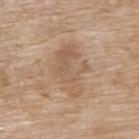TBP lesion metrics — a footprint of about 18 mm², an outline eccentricity of about 0.75 (0 = round, 1 = elongated), and a shape-asymmetry score of about 0.25 (0 = symmetric); a nevus-likeness score of about 0/100 and a lesion-detection confidence of about 100/100
subject — female, in their mid-70s
diameter — about 6 mm
lighting — white-light
image source — ~15 mm tile from a whole-body skin photo
location — the upper back